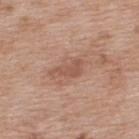{"biopsy_status": "not biopsied; imaged during a skin examination", "lesion_size": {"long_diameter_mm_approx": 4.0}, "image": {"source": "total-body photography crop", "field_of_view_mm": 15}, "lighting": "white-light", "site": "upper back", "automated_metrics": {"cielab_L": 53, "cielab_a": 21, "cielab_b": 29, "vs_skin_darker_L": 9.0, "vs_skin_contrast_norm": 6.0, "nevus_likeness_0_100": 0, "lesion_detection_confidence_0_100": 100}, "patient": {"sex": "female", "age_approx": 40}}